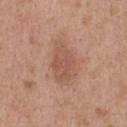– biopsy status · no biopsy performed (imaged during a skin exam)
– lighting · white-light illumination
– image source · 15 mm crop, total-body photography
– location · the head or neck
– size · ≈5 mm
– subject · male, aged 23 to 27
– automated metrics · a footprint of about 12 mm², a shape eccentricity near 0.75, and two-axis asymmetry of about 0.2; a classifier nevus-likeness of about 35/100 and lesion-presence confidence of about 100/100